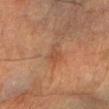This lesion was catalogued during total-body skin photography and was not selected for biopsy. A region of skin cropped from a whole-body photographic capture, roughly 15 mm wide. The lesion is located on the right forearm. The tile uses cross-polarized illumination. The patient is a male aged approximately 85 or older.